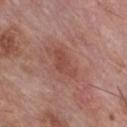{"biopsy_status": "not biopsied; imaged during a skin examination", "image": {"source": "total-body photography crop", "field_of_view_mm": 15}, "lesion_size": {"long_diameter_mm_approx": 4.0}, "patient": {"sex": "male", "age_approx": 60}, "site": "chest", "automated_metrics": {"area_mm2_approx": 4.5, "eccentricity": 0.9, "shape_asymmetry": 0.5, "cielab_L": 47, "cielab_a": 25, "cielab_b": 26, "vs_skin_darker_L": 7.0, "vs_skin_contrast_norm": 5.5, "border_irregularity_0_10": 6.0, "color_variation_0_10": 1.5, "peripheral_color_asymmetry": 0.5, "nevus_likeness_0_100": 5, "lesion_detection_confidence_0_100": 100}, "lighting": "white-light"}